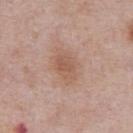A 15 mm close-up tile from a total-body photography series done for melanoma screening. Automated image analysis of the tile measured a footprint of about 9.5 mm² and an outline eccentricity of about 0.8 (0 = round, 1 = elongated). The analysis additionally found a lesion color around L≈57 a*≈20 b*≈28 in CIELAB, about 8 CIELAB-L* units darker than the surrounding skin, and a normalized border contrast of about 6. It also reported a border-irregularity rating of about 2.5/10, a color-variation rating of about 2.5/10, and a peripheral color-asymmetry measure near 1. The software also gave lesion-presence confidence of about 100/100. A male patient, about 75 years old. The tile uses white-light illumination. Longest diameter approximately 4.5 mm. Located on the chest.